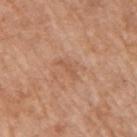A 15 mm close-up extracted from a 3D total-body photography capture.
The total-body-photography lesion software estimated an outline eccentricity of about 0.95 (0 = round, 1 = elongated) and two-axis asymmetry of about 0.5. It also reported a lesion color around L≈56 a*≈22 b*≈33 in CIELAB, a lesion–skin lightness drop of about 6, and a lesion-to-skin contrast of about 4.5 (normalized; higher = more distinct). It also reported a border-irregularity rating of about 5.5/10 and peripheral color asymmetry of about 0. It also reported an automated nevus-likeness rating near 0 out of 100 and a detector confidence of about 100 out of 100 that the crop contains a lesion.
The lesion is located on the arm.
A male subject, aged 73–77.